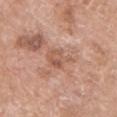This lesion was catalogued during total-body skin photography and was not selected for biopsy.
Automated tile analysis of the lesion measured an area of roughly 6.5 mm², an eccentricity of roughly 0.65, and two-axis asymmetry of about 0.3. It also reported a mean CIELAB color near L≈56 a*≈22 b*≈29. It also reported lesion-presence confidence of about 95/100.
A lesion tile, about 15 mm wide, cut from a 3D total-body photograph.
A female patient roughly 60 years of age.
Approximately 3.5 mm at its widest.
Imaged with white-light lighting.
The lesion is on the chest.